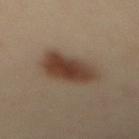Recorded during total-body skin imaging; not selected for excision or biopsy.
A close-up tile cropped from a whole-body skin photograph, about 15 mm across.
On the mid back.
A female subject aged approximately 40.